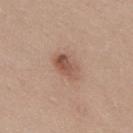Case summary:
* biopsy status: catalogued during a skin exam; not biopsied
* size: ≈3.5 mm
* lighting: white-light
* subject: male, aged around 40
* image: ~15 mm crop, total-body skin-cancer survey
* anatomic site: the upper back